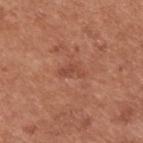Captured during whole-body skin photography for melanoma surveillance; the lesion was not biopsied. The lesion is on the upper back. The total-body-photography lesion software estimated about 7 CIELAB-L* units darker than the surrounding skin and a normalized border contrast of about 5. It also reported a border-irregularity rating of about 6/10, internal color variation of about 0.5 on a 0–10 scale, and a peripheral color-asymmetry measure near 0. And it measured an automated nevus-likeness rating near 0 out of 100. A male subject in their mid- to late 50s. This image is a 15 mm lesion crop taken from a total-body photograph. The lesion's longest dimension is about 3 mm.